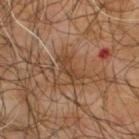Notes:
– workup: imaged on a skin check; not biopsied
– illumination: cross-polarized illumination
– patient: male, approximately 65 years of age
– site: the back
– image-analysis metrics: a footprint of about 5 mm² and a shape-asymmetry score of about 0.3 (0 = symmetric); a classifier nevus-likeness of about 0/100 and a lesion-detection confidence of about 85/100
– image: 15 mm crop, total-body photography